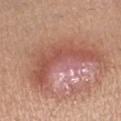Part of a total-body skin-imaging series; this lesion was reviewed on a skin check and was not flagged for biopsy. A lesion tile, about 15 mm wide, cut from a 3D total-body photograph. From the left lower leg. The patient is a female aged around 35.